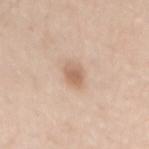Clinical impression: Part of a total-body skin-imaging series; this lesion was reviewed on a skin check and was not flagged for biopsy. Context: The lesion's longest dimension is about 3 mm. From the lower back. Imaged with white-light lighting. A 15 mm close-up extracted from a 3D total-body photography capture. A male subject aged 58 to 62. Automated tile analysis of the lesion measured an average lesion color of about L≈63 a*≈18 b*≈30 (CIELAB), roughly 11 lightness units darker than nearby skin, and a lesion-to-skin contrast of about 7 (normalized; higher = more distinct). And it measured internal color variation of about 3.5 on a 0–10 scale and peripheral color asymmetry of about 1.